Located on the upper back; approximately 2.5 mm at its widest; The lesion-visualizer software estimated a footprint of about 2.5 mm². The analysis additionally found a mean CIELAB color near L≈40 a*≈30 b*≈33, about 5 CIELAB-L* units darker than the surrounding skin, and a normalized lesion–skin contrast near 5. And it measured a nevus-likeness score of about 0/100 and a detector confidence of about 90 out of 100 that the crop contains a lesion; a 15 mm close-up extracted from a 3D total-body photography capture; the subject is a male about 60 years old: 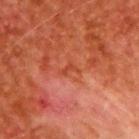<tbp_lesion>
  <diagnosis>
    <histopathology>superficial basal cell carcinoma</histopathology>
    <malignancy>malignant</malignancy>
    <taxonomic_path>Malignant; Malignant adnexal epithelial proliferations - Follicular; Basal cell carcinoma; Basal cell carcinoma, Superficial</taxonomic_path>
  </diagnosis>
</tbp_lesion>